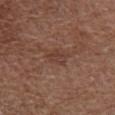Recorded during total-body skin imaging; not selected for excision or biopsy.
The subject is a female roughly 80 years of age.
This image is a 15 mm lesion crop taken from a total-body photograph.
Automated image analysis of the tile measured a mean CIELAB color near L≈39 a*≈19 b*≈25 and roughly 6 lightness units darker than nearby skin. It also reported a border-irregularity rating of about 3/10, a within-lesion color-variation index near 1.5/10, and peripheral color asymmetry of about 0.5.
Captured under white-light illumination.
The lesion's longest dimension is about 2.5 mm.
From the front of the torso.